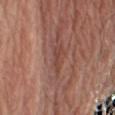Findings:
* workup: catalogued during a skin exam; not biopsied
* image: 15 mm crop, total-body photography
* patient: male, aged around 65
* site: the right upper arm